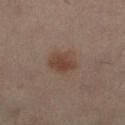• biopsy status — total-body-photography surveillance lesion; no biopsy
• image — ~15 mm crop, total-body skin-cancer survey
• lesion size — ≈3.5 mm
• location — the left leg
• subject — female, approximately 40 years of age